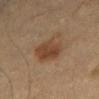workup: total-body-photography surveillance lesion; no biopsy | TBP lesion metrics: an area of roughly 13 mm², a shape eccentricity near 0.2, and a shape-asymmetry score of about 0.2 (0 = symmetric); an average lesion color of about L≈37 a*≈15 b*≈27 (CIELAB), about 8 CIELAB-L* units darker than the surrounding skin, and a normalized lesion–skin contrast near 7.5; border irregularity of about 2 on a 0–10 scale and a peripheral color-asymmetry measure near 1.5 | illumination: cross-polarized | image source: ~15 mm tile from a whole-body skin photo | lesion size: ~4 mm (longest diameter) | site: the right thigh | subject: female, aged 53 to 57.